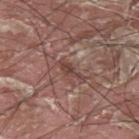The lesion was photographed on a routine skin check and not biopsied; there is no pathology result.
Cropped from a total-body skin-imaging series; the visible field is about 15 mm.
A male patient aged approximately 40.
This is a white-light tile.
Located on the back.
The lesion-visualizer software estimated a mean CIELAB color near L≈42 a*≈20 b*≈22, about 8 CIELAB-L* units darker than the surrounding skin, and a normalized border contrast of about 7. It also reported border irregularity of about 4 on a 0–10 scale, a color-variation rating of about 1.5/10, and peripheral color asymmetry of about 0.5. It also reported a classifier nevus-likeness of about 0/100 and a detector confidence of about 55 out of 100 that the crop contains a lesion.